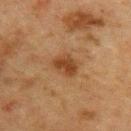acquisition=total-body-photography crop, ~15 mm field of view
TBP lesion metrics=a footprint of about 6.5 mm², an eccentricity of roughly 0.65, and two-axis asymmetry of about 0.2; roughly 8 lightness units darker than nearby skin and a normalized border contrast of about 8; a within-lesion color-variation index near 3/10 and peripheral color asymmetry of about 1; an automated nevus-likeness rating near 80 out of 100
illumination=cross-polarized
subject=female, aged approximately 55
lesion diameter=≈3 mm
location=the upper back